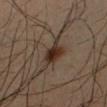The lesion was photographed on a routine skin check and not biopsied; there is no pathology result. Automated image analysis of the tile measured an average lesion color of about L≈29 a*≈15 b*≈23 (CIELAB), about 11 CIELAB-L* units darker than the surrounding skin, and a normalized border contrast of about 11.5. It also reported a classifier nevus-likeness of about 100/100 and a lesion-detection confidence of about 100/100. The tile uses cross-polarized illumination. Located on the arm. A male patient about 40 years old. A 15 mm close-up extracted from a 3D total-body photography capture.